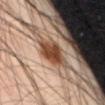Impression: Captured during whole-body skin photography for melanoma surveillance; the lesion was not biopsied. Image and clinical context: A male subject in their mid- to late 40s. The tile uses cross-polarized illumination. An algorithmic analysis of the crop reported a lesion color around L≈43 a*≈20 b*≈29 in CIELAB and a normalized border contrast of about 10.5. And it measured a border-irregularity rating of about 2.5/10 and radial color variation of about 1.5. The analysis additionally found an automated nevus-likeness rating near 100 out of 100. Approximately 5 mm at its widest. On the lower back. A lesion tile, about 15 mm wide, cut from a 3D total-body photograph.